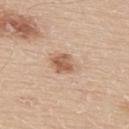<lesion>
  <biopsy_status>not biopsied; imaged during a skin examination</biopsy_status>
  <site>upper back</site>
  <patient>
    <sex>male</sex>
    <age_approx>60</age_approx>
  </patient>
  <lighting>white-light</lighting>
  <automated_metrics>
    <eccentricity>0.7</eccentricity>
    <shape_asymmetry>0.35</shape_asymmetry>
    <lesion_detection_confidence_0_100>100</lesion_detection_confidence_0_100>
  </automated_metrics>
  <image>
    <source>total-body photography crop</source>
    <field_of_view_mm>15</field_of_view_mm>
  </image>
  <lesion_size>
    <long_diameter_mm_approx>5.0</long_diameter_mm_approx>
  </lesion_size>
</lesion>